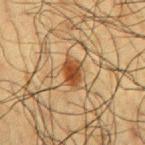Captured during whole-body skin photography for melanoma surveillance; the lesion was not biopsied.
This is a cross-polarized tile.
A lesion tile, about 15 mm wide, cut from a 3D total-body photograph.
Approximately 3 mm at its widest.
On the right upper arm.
A male patient, aged 58 to 62.